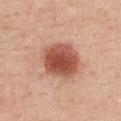The lesion was photographed on a routine skin check and not biopsied; there is no pathology result. A female patient, in their 40s. The recorded lesion diameter is about 5 mm. Cropped from a whole-body photographic skin survey; the tile spans about 15 mm. Imaged with white-light lighting. From the chest. The lesion-visualizer software estimated an average lesion color of about L≈53 a*≈27 b*≈31 (CIELAB), roughly 16 lightness units darker than nearby skin, and a normalized border contrast of about 10.5. The analysis additionally found an automated nevus-likeness rating near 100 out of 100 and lesion-presence confidence of about 100/100.